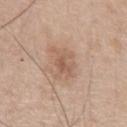<record>
<image>
  <source>total-body photography crop</source>
  <field_of_view_mm>15</field_of_view_mm>
</image>
<lighting>white-light</lighting>
<site>chest</site>
<patient>
  <sex>male</sex>
  <age_approx>65</age_approx>
</patient>
<lesion_size>
  <long_diameter_mm_approx>4.0</long_diameter_mm_approx>
</lesion_size>
</record>